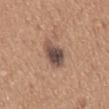The lesion was photographed on a routine skin check and not biopsied; there is no pathology result. A close-up tile cropped from a whole-body skin photograph, about 15 mm across. The lesion is on the mid back. A male patient about 65 years old.